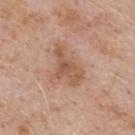workup = imaged on a skin check; not biopsied
tile lighting = white-light illumination
anatomic site = the upper back
size = ≈5 mm
acquisition = ~15 mm crop, total-body skin-cancer survey
image-analysis metrics = roughly 9 lightness units darker than nearby skin; a border-irregularity rating of about 5/10 and peripheral color asymmetry of about 1; a classifier nevus-likeness of about 0/100 and a detector confidence of about 100 out of 100 that the crop contains a lesion
subject = male, aged 58 to 62